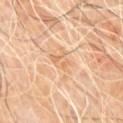Captured during whole-body skin photography for melanoma surveillance; the lesion was not biopsied. The lesion is on the upper back. Measured at roughly 3 mm in maximum diameter. The lesion-visualizer software estimated an area of roughly 4 mm² and an outline eccentricity of about 0.65 (0 = round, 1 = elongated). The software also gave a classifier nevus-likeness of about 0/100 and a lesion-detection confidence of about 95/100. A male subject, aged 58 to 62. Imaged with cross-polarized lighting. A lesion tile, about 15 mm wide, cut from a 3D total-body photograph.